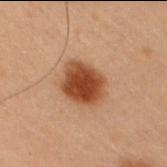notes: imaged on a skin check; not biopsied | site: the right upper arm | image source: ~15 mm crop, total-body skin-cancer survey | subject: male, aged 53 to 57 | tile lighting: cross-polarized | automated metrics: a symmetry-axis asymmetry near 0.15 | size: ~4.5 mm (longest diameter).